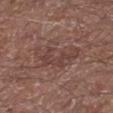No biopsy was performed on this lesion — it was imaged during a full skin examination and was not determined to be concerning.
A 15 mm close-up extracted from a 3D total-body photography capture.
A male patient approximately 55 years of age.
This is a white-light tile.
From the left lower leg.
The lesion-visualizer software estimated a lesion–skin lightness drop of about 7. It also reported a nevus-likeness score of about 0/100 and a detector confidence of about 85 out of 100 that the crop contains a lesion.
About 6 mm across.